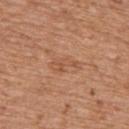Part of a total-body skin-imaging series; this lesion was reviewed on a skin check and was not flagged for biopsy. Approximately 3.5 mm at its widest. The patient is a male aged 63 to 67. Imaged with white-light lighting. A region of skin cropped from a whole-body photographic capture, roughly 15 mm wide. On the upper back.